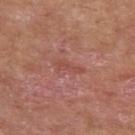notes — total-body-photography surveillance lesion; no biopsy
image-analysis metrics — an average lesion color of about L≈49 a*≈26 b*≈27 (CIELAB) and a lesion-to-skin contrast of about 4.5 (normalized; higher = more distinct); a border-irregularity index near 4.5/10
subject — male, about 65 years old
site — the left upper arm
lesion diameter — about 3.5 mm
image — ~15 mm tile from a whole-body skin photo